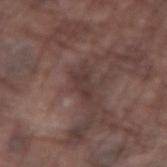biopsy status = total-body-photography surveillance lesion; no biopsy
site = the arm
lesion diameter = ≈3.5 mm
image = ~15 mm crop, total-body skin-cancer survey
patient = male, aged around 75